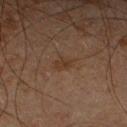<case>
  <biopsy_status>not biopsied; imaged during a skin examination</biopsy_status>
  <image>
    <source>total-body photography crop</source>
    <field_of_view_mm>15</field_of_view_mm>
  </image>
  <lesion_size>
    <long_diameter_mm_approx>2.5</long_diameter_mm_approx>
  </lesion_size>
  <lighting>cross-polarized</lighting>
  <patient>
    <sex>male</sex>
    <age_approx>65</age_approx>
  </patient>
  <site>right forearm</site>
  <automated_metrics>
    <area_mm2_approx>3.0</area_mm2_approx>
    <eccentricity>0.8</eccentricity>
    <shape_asymmetry>0.3</shape_asymmetry>
    <border_irregularity_0_10>2.5</border_irregularity_0_10>
    <nevus_likeness_0_100>0</nevus_likeness_0_100>
    <lesion_detection_confidence_0_100>100</lesion_detection_confidence_0_100>
  </automated_metrics>
</case>